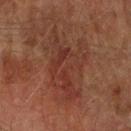Q: Was this lesion biopsied?
A: imaged on a skin check; not biopsied
Q: How was this image acquired?
A: total-body-photography crop, ~15 mm field of view
Q: Who is the patient?
A: male, in their mid- to late 70s
Q: Lesion location?
A: the arm
Q: What did automated image analysis measure?
A: an area of roughly 20 mm², an eccentricity of roughly 0.8, and a symmetry-axis asymmetry near 0.55; a mean CIELAB color near L≈28 a*≈20 b*≈22, a lesion–skin lightness drop of about 5, and a normalized lesion–skin contrast near 6; internal color variation of about 4 on a 0–10 scale and radial color variation of about 1; an automated nevus-likeness rating near 0 out of 100 and a lesion-detection confidence of about 100/100